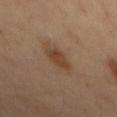notes: no biopsy performed (imaged during a skin exam)
automated metrics: an average lesion color of about L≈40 a*≈18 b*≈29 (CIELAB), roughly 8 lightness units darker than nearby skin, and a lesion-to-skin contrast of about 7.5 (normalized; higher = more distinct); an automated nevus-likeness rating near 75 out of 100 and a detector confidence of about 100 out of 100 that the crop contains a lesion
anatomic site: the back
tile lighting: cross-polarized
lesion size: ≈3 mm
subject: male, about 55 years old
acquisition: 15 mm crop, total-body photography